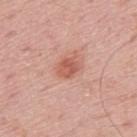Recorded during total-body skin imaging; not selected for excision or biopsy. Imaged with white-light lighting. A 15 mm crop from a total-body photograph taken for skin-cancer surveillance. A male subject, approximately 50 years of age. On the upper back.